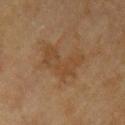follow-up: imaged on a skin check; not biopsied
tile lighting: cross-polarized
site: the left upper arm
subject: female, aged around 80
size: ~6 mm (longest diameter)
image source: total-body-photography crop, ~15 mm field of view
TBP lesion metrics: a mean CIELAB color near L≈38 a*≈15 b*≈29 and a lesion–skin lightness drop of about 5; a nevus-likeness score of about 0/100 and a lesion-detection confidence of about 100/100